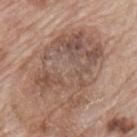lighting: white-light
image:
  source: total-body photography crop
  field_of_view_mm: 15
patient:
  sex: male
  age_approx: 70
automated_metrics:
  area_mm2_approx: 46.0
  eccentricity: 0.7
  shape_asymmetry: 0.25
  border_irregularity_0_10: 4.0
  color_variation_0_10: 7.0
  nevus_likeness_0_100: 0
  lesion_detection_confidence_0_100: 100
site: mid back